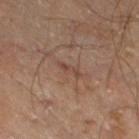Q: Is there a histopathology result?
A: total-body-photography surveillance lesion; no biopsy
Q: Where on the body is the lesion?
A: the right thigh
Q: Patient demographics?
A: male, aged 68–72
Q: What kind of image is this?
A: ~15 mm crop, total-body skin-cancer survey
Q: How was the tile lit?
A: cross-polarized illumination